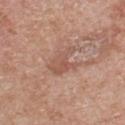workup=imaged on a skin check; not biopsied | illumination=white-light | lesion diameter=about 4 mm | image source=~15 mm tile from a whole-body skin photo | subject=male, about 70 years old | body site=the front of the torso.